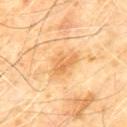Impression: No biopsy was performed on this lesion — it was imaged during a full skin examination and was not determined to be concerning. Acquisition and patient details: This image is a 15 mm lesion crop taken from a total-body photograph. A male subject, approximately 60 years of age. Located on the chest.